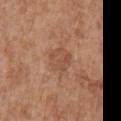Part of a total-body skin-imaging series; this lesion was reviewed on a skin check and was not flagged for biopsy. The lesion's longest dimension is about 3.5 mm. An algorithmic analysis of the crop reported roughly 7 lightness units darker than nearby skin and a lesion-to-skin contrast of about 5 (normalized; higher = more distinct). This is a white-light tile. A region of skin cropped from a whole-body photographic capture, roughly 15 mm wide. A female patient aged 73 to 77. Located on the right upper arm.